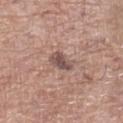A male subject, aged 68–72. The lesion is located on the leg. This is a white-light tile. A roughly 15 mm field-of-view crop from a total-body skin photograph. An algorithmic analysis of the crop reported an eccentricity of roughly 0.85 and a symmetry-axis asymmetry near 0.3. The analysis additionally found an average lesion color of about L≈51 a*≈17 b*≈22 (CIELAB), about 11 CIELAB-L* units darker than the surrounding skin, and a normalized lesion–skin contrast near 8. The analysis additionally found a border-irregularity index near 3/10, internal color variation of about 3 on a 0–10 scale, and peripheral color asymmetry of about 1. The analysis additionally found a detector confidence of about 100 out of 100 that the crop contains a lesion. About 3.5 mm across.